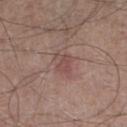notes=total-body-photography surveillance lesion; no biopsy | patient=male, aged 63–67 | size=about 3 mm | image=~15 mm tile from a whole-body skin photo | location=the right lower leg.